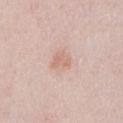Assessment: The lesion was tiled from a total-body skin photograph and was not biopsied. Clinical summary: Located on the front of the torso. A 15 mm crop from a total-body photograph taken for skin-cancer surveillance. The patient is a male aged around 25.